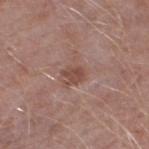Impression:
The lesion was photographed on a routine skin check and not biopsied; there is no pathology result.
Context:
Cropped from a whole-body photographic skin survey; the tile spans about 15 mm. The patient is a male about 50 years old. The lesion is on the left thigh.